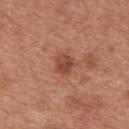Acquisition and patient details: The lesion is on the abdomen. Automated image analysis of the tile measured a footprint of about 5 mm², an outline eccentricity of about 0.5 (0 = round, 1 = elongated), and a symmetry-axis asymmetry near 0.2. And it measured a border-irregularity index near 2/10, a color-variation rating of about 4/10, and peripheral color asymmetry of about 1.5. Imaged with white-light lighting. A 15 mm close-up tile from a total-body photography series done for melanoma screening. The patient is a male aged around 65.